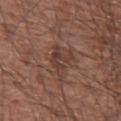Captured during whole-body skin photography for melanoma surveillance; the lesion was not biopsied.
A male patient aged 63 to 67.
From the right upper arm.
A lesion tile, about 15 mm wide, cut from a 3D total-body photograph.
Measured at roughly 3.5 mm in maximum diameter.
Automated image analysis of the tile measured a lesion color around L≈39 a*≈20 b*≈23 in CIELAB, a lesion–skin lightness drop of about 7, and a lesion-to-skin contrast of about 6.5 (normalized; higher = more distinct). The software also gave border irregularity of about 7 on a 0–10 scale, a within-lesion color-variation index near 3/10, and radial color variation of about 1. The software also gave a nevus-likeness score of about 0/100 and a lesion-detection confidence of about 100/100.
This is a white-light tile.